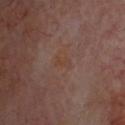This lesion was catalogued during total-body skin photography and was not selected for biopsy. The lesion-visualizer software estimated a footprint of about 2 mm², a shape eccentricity near 0.95, and a symmetry-axis asymmetry near 0.4. The analysis additionally found a mean CIELAB color near L≈39 a*≈18 b*≈25, a lesion–skin lightness drop of about 3, and a normalized lesion–skin contrast near 5. The patient is in their mid-60s. A lesion tile, about 15 mm wide, cut from a 3D total-body photograph. The tile uses cross-polarized illumination. Located on the head or neck. The lesion's longest dimension is about 2.5 mm.